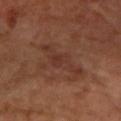Clinical summary:
An algorithmic analysis of the crop reported a footprint of about 11 mm², a shape eccentricity near 0.95, and a shape-asymmetry score of about 0.4 (0 = symmetric). It also reported an automated nevus-likeness rating near 0 out of 100. Cropped from a whole-body photographic skin survey; the tile spans about 15 mm. A female subject, roughly 70 years of age. About 6.5 mm across. Located on the left forearm.